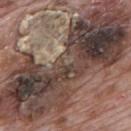Q: Is there a histopathology result?
A: catalogued during a skin exam; not biopsied
Q: How was the tile lit?
A: white-light illumination
Q: Automated lesion metrics?
A: a footprint of about 120 mm², an eccentricity of roughly 0.95, and a shape-asymmetry score of about 0.25 (0 = symmetric); border irregularity of about 7 on a 0–10 scale, a color-variation rating of about 10/10, and peripheral color asymmetry of about 4; a classifier nevus-likeness of about 0/100 and lesion-presence confidence of about 85/100
Q: Where on the body is the lesion?
A: the mid back
Q: What are the patient's age and sex?
A: male, aged 68 to 72
Q: What kind of image is this?
A: ~15 mm crop, total-body skin-cancer survey
Q: How large is the lesion?
A: about 20.5 mm